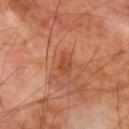Located on the left forearm. Captured under cross-polarized illumination. Automated image analysis of the tile measured an area of roughly 3.5 mm². It also reported an automated nevus-likeness rating near 0 out of 100 and a detector confidence of about 100 out of 100 that the crop contains a lesion. Longest diameter approximately 3 mm. A male subject, aged 68 to 72. A 15 mm crop from a total-body photograph taken for skin-cancer surveillance.